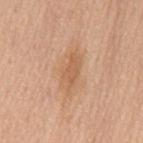biopsy_status: not biopsied; imaged during a skin examination
image:
  source: total-body photography crop
  field_of_view_mm: 15
lesion_size:
  long_diameter_mm_approx: 5.0
lighting: white-light
automated_metrics:
  border_irregularity_0_10: 4.0
  color_variation_0_10: 2.0
patient:
  sex: female
  age_approx: 60
site: mid back